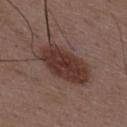Clinical impression: No biopsy was performed on this lesion — it was imaged during a full skin examination and was not determined to be concerning. Image and clinical context: A roughly 15 mm field-of-view crop from a total-body skin photograph. Located on the mid back. A male subject, aged 48 to 52. The lesion's longest dimension is about 7.5 mm. The tile uses white-light illumination. The lesion-visualizer software estimated an area of roughly 23 mm², an outline eccentricity of about 0.85 (0 = round, 1 = elongated), and a shape-asymmetry score of about 0.2 (0 = symmetric). And it measured a mean CIELAB color near L≈35 a*≈18 b*≈22, a lesion–skin lightness drop of about 11, and a normalized lesion–skin contrast near 9.5. It also reported a border-irregularity index near 3/10, internal color variation of about 3.5 on a 0–10 scale, and peripheral color asymmetry of about 1. It also reported a lesion-detection confidence of about 100/100.